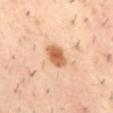Impression:
Part of a total-body skin-imaging series; this lesion was reviewed on a skin check and was not flagged for biopsy.
Clinical summary:
The lesion's longest dimension is about 3 mm. A 15 mm close-up tile from a total-body photography series done for melanoma screening. Imaged with cross-polarized lighting. Located on the mid back. Automated tile analysis of the lesion measured a shape eccentricity near 0.7 and two-axis asymmetry of about 0.2. The software also gave a mean CIELAB color near L≈61 a*≈25 b*≈37, a lesion–skin lightness drop of about 15, and a normalized border contrast of about 9.5. And it measured a border-irregularity rating of about 2/10, a within-lesion color-variation index near 4/10, and peripheral color asymmetry of about 1.5. The patient is a male aged 38 to 42.